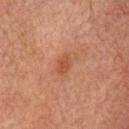Image and clinical context: A close-up tile cropped from a whole-body skin photograph, about 15 mm across. Located on the head or neck. An algorithmic analysis of the crop reported a lesion color around L≈42 a*≈23 b*≈30 in CIELAB and a lesion–skin lightness drop of about 7. The analysis additionally found border irregularity of about 2 on a 0–10 scale, a within-lesion color-variation index near 1.5/10, and radial color variation of about 0.5. And it measured an automated nevus-likeness rating near 35 out of 100. Longest diameter approximately 2.5 mm. A male patient, aged around 75. The tile uses cross-polarized illumination.